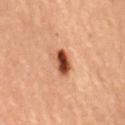Impression:
Imaged during a routine full-body skin examination; the lesion was not biopsied and no histopathology is available.
Image and clinical context:
The patient is a female approximately 70 years of age. Captured under cross-polarized illumination. A roughly 15 mm field-of-view crop from a total-body skin photograph. Automated tile analysis of the lesion measured a lesion area of about 5.5 mm², an outline eccentricity of about 0.85 (0 = round, 1 = elongated), and a shape-asymmetry score of about 0.3 (0 = symmetric). It also reported an average lesion color of about L≈47 a*≈27 b*≈35 (CIELAB), roughly 20 lightness units darker than nearby skin, and a normalized border contrast of about 13. The software also gave an automated nevus-likeness rating near 100 out of 100. On the mid back. Longest diameter approximately 3.5 mm.